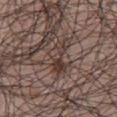| key | value |
|---|---|
| follow-up | imaged on a skin check; not biopsied |
| patient | male, in their 50s |
| body site | the chest |
| image source | ~15 mm tile from a whole-body skin photo |